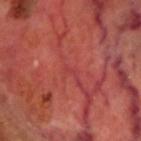The lesion was tiled from a total-body skin photograph and was not biopsied. The tile uses cross-polarized illumination. The lesion is on the head or neck. Measured at roughly 1.5 mm in maximum diameter. A male patient, roughly 70 years of age. The lesion-visualizer software estimated an area of roughly 0.5 mm², a shape eccentricity near 0.95, and a shape-asymmetry score of about 0.6 (0 = symmetric). The analysis additionally found a normalized border contrast of about 3.5. It also reported a classifier nevus-likeness of about 0/100. A region of skin cropped from a whole-body photographic capture, roughly 15 mm wide.